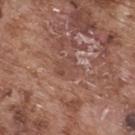Part of a total-body skin-imaging series; this lesion was reviewed on a skin check and was not flagged for biopsy. On the upper back. About 3 mm across. The total-body-photography lesion software estimated a within-lesion color-variation index near 2.5/10 and peripheral color asymmetry of about 1. A male subject, approximately 75 years of age. A lesion tile, about 15 mm wide, cut from a 3D total-body photograph. The tile uses white-light illumination.